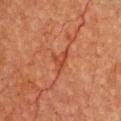{"biopsy_status": "not biopsied; imaged during a skin examination"}